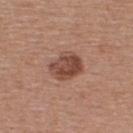| key | value |
|---|---|
| lighting | white-light illumination |
| automated metrics | a mean CIELAB color near L≈46 a*≈22 b*≈27 and a lesion-to-skin contrast of about 9 (normalized; higher = more distinct); a border-irregularity index near 1.5/10, a within-lesion color-variation index near 4.5/10, and peripheral color asymmetry of about 1.5 |
| imaging modality | 15 mm crop, total-body photography |
| location | the upper back |
| size | ≈3.5 mm |
| patient | male, in their mid-50s |